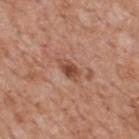Recorded during total-body skin imaging; not selected for excision or biopsy.
The recorded lesion diameter is about 3.5 mm.
On the mid back.
The tile uses white-light illumination.
A roughly 15 mm field-of-view crop from a total-body skin photograph.
The patient is a male aged 63–67.